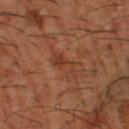Part of a total-body skin-imaging series; this lesion was reviewed on a skin check and was not flagged for biopsy.
The lesion is located on the left thigh.
A 15 mm crop from a total-body photograph taken for skin-cancer surveillance.
About 4.5 mm across.
The lesion-visualizer software estimated a footprint of about 5 mm² and a shape-asymmetry score of about 0.5 (0 = symmetric). It also reported a lesion color around L≈39 a*≈24 b*≈32 in CIELAB and roughly 6 lightness units darker than nearby skin. And it measured a border-irregularity index near 7.5/10 and a peripheral color-asymmetry measure near 0.
Captured under cross-polarized illumination.
A male patient, aged around 65.